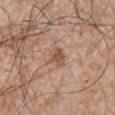location = the chest
subject = male, approximately 65 years of age
image = ~15 mm tile from a whole-body skin photo